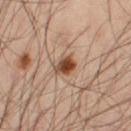Captured during whole-body skin photography for melanoma surveillance; the lesion was not biopsied.
The lesion is located on the front of the torso.
The patient is a male roughly 50 years of age.
Measured at roughly 2.5 mm in maximum diameter.
The total-body-photography lesion software estimated a mean CIELAB color near L≈47 a*≈21 b*≈31, a lesion–skin lightness drop of about 15, and a normalized border contrast of about 11. And it measured a border-irregularity rating of about 2.5/10, internal color variation of about 7 on a 0–10 scale, and a peripheral color-asymmetry measure near 2. It also reported a detector confidence of about 100 out of 100 that the crop contains a lesion.
Imaged with cross-polarized lighting.
A roughly 15 mm field-of-view crop from a total-body skin photograph.